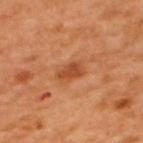Imaged during a routine full-body skin examination; the lesion was not biopsied and no histopathology is available.
A male patient aged approximately 50.
A roughly 15 mm field-of-view crop from a total-body skin photograph.
Located on the upper back.